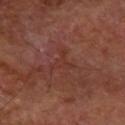{"site": "right forearm", "image": {"source": "total-body photography crop", "field_of_view_mm": 15}, "patient": {"sex": "male", "age_approx": 60}}